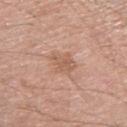  biopsy_status: not biopsied; imaged during a skin examination
  site: right upper arm
  lesion_size:
    long_diameter_mm_approx: 3.0
  image:
    source: total-body photography crop
    field_of_view_mm: 15
  lighting: white-light
  patient:
    sex: male
    age_approx: 60